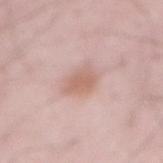{
  "biopsy_status": "not biopsied; imaged during a skin examination",
  "image": {
    "source": "total-body photography crop",
    "field_of_view_mm": 15
  },
  "patient": {
    "sex": "male",
    "age_approx": 65
  },
  "site": "abdomen",
  "automated_metrics": {
    "area_mm2_approx": 6.5,
    "eccentricity": 0.4,
    "shape_asymmetry": 0.25
  },
  "lesion_size": {
    "long_diameter_mm_approx": 3.0
  }
}